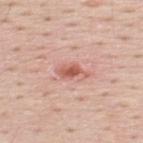Impression: Imaged during a routine full-body skin examination; the lesion was not biopsied and no histopathology is available. Context: Measured at roughly 4 mm in maximum diameter. Imaged with white-light lighting. The subject is a male aged approximately 50. On the back. Automated image analysis of the tile measured a lesion-to-skin contrast of about 7.5 (normalized; higher = more distinct). The software also gave internal color variation of about 3 on a 0–10 scale and peripheral color asymmetry of about 0.5. A 15 mm close-up extracted from a 3D total-body photography capture.